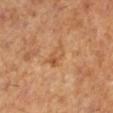This lesion was catalogued during total-body skin photography and was not selected for biopsy. Imaged with cross-polarized lighting. A female patient, aged 63 to 67. The lesion is located on the left lower leg. Cropped from a total-body skin-imaging series; the visible field is about 15 mm.